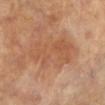Case summary:
- follow-up · total-body-photography surveillance lesion; no biopsy
- image · 15 mm crop, total-body photography
- image-analysis metrics · a shape eccentricity near 0.9 and a shape-asymmetry score of about 0.3 (0 = symmetric); a lesion–skin lightness drop of about 6 and a lesion-to-skin contrast of about 5 (normalized; higher = more distinct); a within-lesion color-variation index near 3/10 and radial color variation of about 1
- anatomic site · the right leg
- illumination · cross-polarized
- subject · female, aged around 65